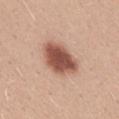Recorded during total-body skin imaging; not selected for excision or biopsy. The lesion-visualizer software estimated a normalized border contrast of about 11. The analysis additionally found a border-irregularity rating of about 2/10, internal color variation of about 4.5 on a 0–10 scale, and radial color variation of about 1.5. Longest diameter approximately 5.5 mm. Located on the back. Cropped from a total-body skin-imaging series; the visible field is about 15 mm. Captured under white-light illumination. A female subject, roughly 25 years of age.